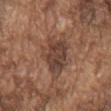biopsy_status: not biopsied; imaged during a skin examination
image:
  source: total-body photography crop
  field_of_view_mm: 15
site: chest
lighting: white-light
patient:
  sex: male
  age_approx: 75
lesion_size:
  long_diameter_mm_approx: 6.5